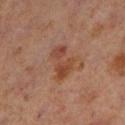Assessment:
Part of a total-body skin-imaging series; this lesion was reviewed on a skin check and was not flagged for biopsy.
Image and clinical context:
From the left lower leg. A male patient aged approximately 60. Cropped from a total-body skin-imaging series; the visible field is about 15 mm.